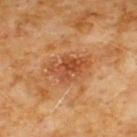Assessment: No biopsy was performed on this lesion — it was imaged during a full skin examination and was not determined to be concerning. Clinical summary: Measured at roughly 4.5 mm in maximum diameter. A male subject, aged around 60. The total-body-photography lesion software estimated an area of roughly 10 mm², an eccentricity of roughly 0.8, and a symmetry-axis asymmetry near 0.3. It also reported an average lesion color of about L≈48 a*≈27 b*≈38 (CIELAB) and about 10 CIELAB-L* units darker than the surrounding skin. It also reported internal color variation of about 5 on a 0–10 scale and a peripheral color-asymmetry measure near 1.5. It also reported a classifier nevus-likeness of about 25/100 and a detector confidence of about 100 out of 100 that the crop contains a lesion. The lesion is located on the chest. A roughly 15 mm field-of-view crop from a total-body skin photograph. Captured under cross-polarized illumination.